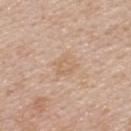<case>
<automated_metrics>
  <area_mm2_approx>6.0</area_mm2_approx>
  <eccentricity>0.35</eccentricity>
  <cielab_L>64</cielab_L>
  <cielab_a>17</cielab_a>
  <cielab_b>32</cielab_b>
  <vs_skin_darker_L>6.0</vs_skin_darker_L>
  <border_irregularity_0_10>2.0</border_irregularity_0_10>
  <color_variation_0_10>2.0</color_variation_0_10>
  <nevus_likeness_0_100>0</nevus_likeness_0_100>
  <lesion_detection_confidence_0_100>100</lesion_detection_confidence_0_100>
</automated_metrics>
<site>back</site>
<lighting>white-light</lighting>
<lesion_size>
  <long_diameter_mm_approx>3.0</long_diameter_mm_approx>
</lesion_size>
<patient>
  <sex>female</sex>
  <age_approx>45</age_approx>
</patient>
<image>
  <source>total-body photography crop</source>
  <field_of_view_mm>15</field_of_view_mm>
</image>
</case>